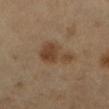<case>
<patient>
  <sex>female</sex>
  <age_approx>60</age_approx>
</patient>
<lesion_size>
  <long_diameter_mm_approx>4.5</long_diameter_mm_approx>
</lesion_size>
<site>left lower leg</site>
<image>
  <source>total-body photography crop</source>
  <field_of_view_mm>15</field_of_view_mm>
</image>
</case>